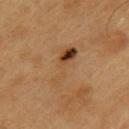  biopsy_status: not biopsied; imaged during a skin examination
  patient:
    sex: male
    age_approx: 60
  image:
    source: total-body photography crop
    field_of_view_mm: 15
  site: upper back